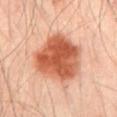Q: Was a biopsy performed?
A: total-body-photography surveillance lesion; no biopsy
Q: What is the anatomic site?
A: the abdomen
Q: How was this image acquired?
A: ~15 mm tile from a whole-body skin photo
Q: Patient demographics?
A: male, in their mid-60s
Q: How large is the lesion?
A: about 6 mm
Q: Automated lesion metrics?
A: an average lesion color of about L≈56 a*≈30 b*≈36 (CIELAB), a lesion–skin lightness drop of about 17, and a normalized lesion–skin contrast near 11; a classifier nevus-likeness of about 100/100